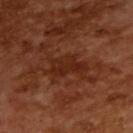Q: Is there a histopathology result?
A: no biopsy performed (imaged during a skin exam)
Q: How was this image acquired?
A: 15 mm crop, total-body photography
Q: Patient demographics?
A: male, in their mid-60s